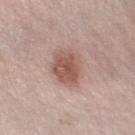{
  "biopsy_status": "not biopsied; imaged during a skin examination",
  "patient": {
    "sex": "female",
    "age_approx": 30
  },
  "image": {
    "source": "total-body photography crop",
    "field_of_view_mm": 15
  },
  "lighting": "white-light",
  "automated_metrics": {
    "area_mm2_approx": 12.0,
    "eccentricity": 0.6,
    "shape_asymmetry": 0.15,
    "border_irregularity_0_10": 1.5,
    "color_variation_0_10": 3.5,
    "peripheral_color_asymmetry": 1.0
  },
  "site": "leg",
  "lesion_size": {
    "long_diameter_mm_approx": 4.5
  }
}